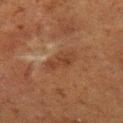follow-up: no biopsy performed (imaged during a skin exam) | image: 15 mm crop, total-body photography | illumination: cross-polarized | location: the left thigh | patient: female, aged around 50 | TBP lesion metrics: a lesion area of about 6 mm² and a symmetry-axis asymmetry near 0.25; a lesion color around L≈33 a*≈19 b*≈27 in CIELAB and a normalized border contrast of about 6; a border-irregularity rating of about 3.5/10, internal color variation of about 2.5 on a 0–10 scale, and radial color variation of about 1; a detector confidence of about 100 out of 100 that the crop contains a lesion | lesion size: ≈4 mm.